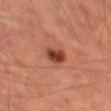notes = imaged on a skin check; not biopsied.